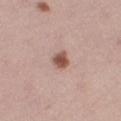The lesion was tiled from a total-body skin photograph and was not biopsied. A female subject in their mid-20s. On the right thigh. Approximately 2.5 mm at its widest. Captured under white-light illumination. Cropped from a whole-body photographic skin survey; the tile spans about 15 mm.